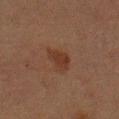| field | value |
|---|---|
| follow-up | no biopsy performed (imaged during a skin exam) |
| site | the left upper arm |
| lesion size | ~3 mm (longest diameter) |
| acquisition | total-body-photography crop, ~15 mm field of view |
| subject | female, roughly 55 years of age |
| TBP lesion metrics | an area of roughly 5 mm² and two-axis asymmetry of about 0.3; an average lesion color of about L≈28 a*≈18 b*≈24 (CIELAB), roughly 6 lightness units darker than nearby skin, and a lesion-to-skin contrast of about 7 (normalized; higher = more distinct); a nevus-likeness score of about 45/100 and a detector confidence of about 100 out of 100 that the crop contains a lesion |